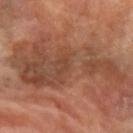Impression:
The lesion was tiled from a total-body skin photograph and was not biopsied.
Image and clinical context:
The tile uses cross-polarized illumination. The recorded lesion diameter is about 14 mm. A female subject about 65 years old. The lesion is located on the left forearm. A 15 mm close-up extracted from a 3D total-body photography capture.